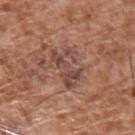Q: Is there a histopathology result?
A: catalogued during a skin exam; not biopsied
Q: Illumination type?
A: white-light
Q: What kind of image is this?
A: ~15 mm crop, total-body skin-cancer survey
Q: What are the patient's age and sex?
A: male, approximately 75 years of age
Q: Lesion location?
A: the upper back
Q: How large is the lesion?
A: ≈5 mm